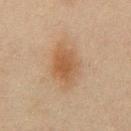biopsy_status: not biopsied; imaged during a skin examination
patient:
  sex: male
  age_approx: 45
lesion_size:
  long_diameter_mm_approx: 5.5
image:
  source: total-body photography crop
  field_of_view_mm: 15
site: abdomen
lighting: cross-polarized